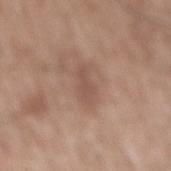Notes:
– biopsy status: no biopsy performed (imaged during a skin exam)
– site: the mid back
– image: ~15 mm crop, total-body skin-cancer survey
– automated metrics: border irregularity of about 4 on a 0–10 scale, internal color variation of about 1 on a 0–10 scale, and a peripheral color-asymmetry measure near 0
– subject: male, in their 60s